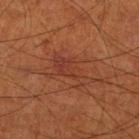Captured during whole-body skin photography for melanoma surveillance; the lesion was not biopsied.
This image is a 15 mm lesion crop taken from a total-body photograph.
A male patient, about 70 years old.
The lesion is located on the right thigh.